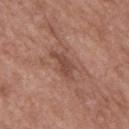Impression:
This lesion was catalogued during total-body skin photography and was not selected for biopsy.
Clinical summary:
Measured at roughly 4.5 mm in maximum diameter. A 15 mm crop from a total-body photograph taken for skin-cancer surveillance. The patient is a male about 50 years old. Captured under white-light illumination. From the back.